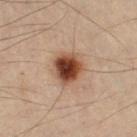<tbp_lesion>
<biopsy_status>not biopsied; imaged during a skin examination</biopsy_status>
</tbp_lesion>